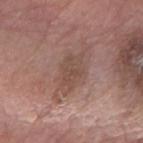No biopsy was performed on this lesion — it was imaged during a full skin examination and was not determined to be concerning.
Automated tile analysis of the lesion measured a lesion color around L≈48 a*≈18 b*≈24 in CIELAB, roughly 7 lightness units darker than nearby skin, and a normalized border contrast of about 5.5. The analysis additionally found a border-irregularity rating of about 3.5/10, internal color variation of about 2 on a 0–10 scale, and a peripheral color-asymmetry measure near 1.
A male subject, aged 58–62.
About 4 mm across.
A close-up tile cropped from a whole-body skin photograph, about 15 mm across.
Captured under white-light illumination.
Located on the right forearm.